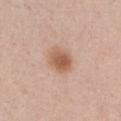{
  "biopsy_status": "not biopsied; imaged during a skin examination",
  "lesion_size": {
    "long_diameter_mm_approx": 3.5
  },
  "lighting": "white-light",
  "automated_metrics": {
    "area_mm2_approx": 7.5,
    "eccentricity": 0.6,
    "shape_asymmetry": 0.2,
    "vs_skin_contrast_norm": 8.5,
    "nevus_likeness_0_100": 95,
    "lesion_detection_confidence_0_100": 100
  },
  "image": {
    "source": "total-body photography crop",
    "field_of_view_mm": 15
  },
  "patient": {
    "sex": "female",
    "age_approx": 40
  },
  "site": "chest"
}